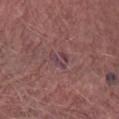workup: total-body-photography surveillance lesion; no biopsy | tile lighting: white-light illumination | location: the abdomen | subject: female, aged approximately 65 | image: ~15 mm crop, total-body skin-cancer survey | image-analysis metrics: a lesion area of about 4 mm²; about 6 CIELAB-L* units darker than the surrounding skin and a normalized lesion–skin contrast near 7; a border-irregularity index near 5.5/10 and peripheral color asymmetry of about 0.